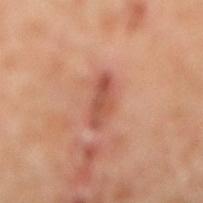Impression:
Part of a total-body skin-imaging series; this lesion was reviewed on a skin check and was not flagged for biopsy.
Image and clinical context:
Located on the right lower leg. Automated image analysis of the tile measured an average lesion color of about L≈51 a*≈26 b*≈30 (CIELAB) and a normalized border contrast of about 7. The tile uses cross-polarized illumination. The lesion's longest dimension is about 4.5 mm. A male subject in their mid-50s. A lesion tile, about 15 mm wide, cut from a 3D total-body photograph.